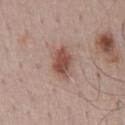Imaged during a routine full-body skin examination; the lesion was not biopsied and no histopathology is available. This image is a 15 mm lesion crop taken from a total-body photograph. The lesion is on the mid back. The subject is a male aged 48 to 52.